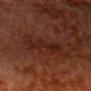Notes:
– notes · no biopsy performed (imaged during a skin exam)
– site · the head or neck
– subject · male, aged approximately 80
– illumination · cross-polarized
– lesion diameter · ≈4.5 mm
– imaging modality · 15 mm crop, total-body photography
– TBP lesion metrics · a border-irregularity rating of about 8/10 and a within-lesion color-variation index near 1.5/10; a classifier nevus-likeness of about 5/100